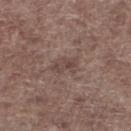Assessment: Captured during whole-body skin photography for melanoma surveillance; the lesion was not biopsied. Context: About 3 mm across. The lesion is on the right lower leg. Cropped from a total-body skin-imaging series; the visible field is about 15 mm. Automated image analysis of the tile measured a shape eccentricity near 0.85. It also reported an average lesion color of about L≈44 a*≈16 b*≈20 (CIELAB) and a normalized border contrast of about 5.5. And it measured an automated nevus-likeness rating near 0 out of 100. A male subject, in their 70s. Captured under white-light illumination.